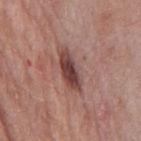notes=no biopsy performed (imaged during a skin exam)
location=the chest
image=total-body-photography crop, ~15 mm field of view
image-analysis metrics=a classifier nevus-likeness of about 15/100 and a detector confidence of about 100 out of 100 that the crop contains a lesion
subject=male, aged around 55
tile lighting=white-light illumination
size=~5.5 mm (longest diameter)